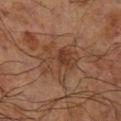The patient is a male aged around 70.
The recorded lesion diameter is about 5.5 mm.
An algorithmic analysis of the crop reported a footprint of about 12 mm². And it measured a mean CIELAB color near L≈31 a*≈16 b*≈25, a lesion–skin lightness drop of about 6, and a normalized lesion–skin contrast near 6. And it measured a nevus-likeness score of about 0/100 and a lesion-detection confidence of about 100/100.
Located on the right lower leg.
A 15 mm close-up extracted from a 3D total-body photography capture.